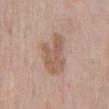  biopsy_status: not biopsied; imaged during a skin examination
  image:
    source: total-body photography crop
    field_of_view_mm: 15
  patient:
    sex: male
    age_approx: 60
  site: abdomen
  automated_metrics:
    cielab_L: 57
    cielab_a: 17
    cielab_b: 28
    vs_skin_darker_L: 9.0
    border_irregularity_0_10: 4.0
    color_variation_0_10: 3.0
    peripheral_color_asymmetry: 1.0
  lesion_size:
    long_diameter_mm_approx: 5.5